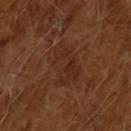Notes:
- workup — total-body-photography surveillance lesion; no biopsy
- patient — male, in their mid-60s
- lesion size — ≈5 mm
- image — 15 mm crop, total-body photography